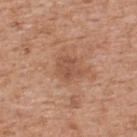Assessment: Imaged during a routine full-body skin examination; the lesion was not biopsied and no histopathology is available. Background: Automated image analysis of the tile measured a lesion color around L≈52 a*≈22 b*≈31 in CIELAB, a lesion–skin lightness drop of about 8, and a lesion-to-skin contrast of about 5.5 (normalized; higher = more distinct). It also reported a border-irregularity rating of about 3.5/10, internal color variation of about 2.5 on a 0–10 scale, and radial color variation of about 1. The software also gave a nevus-likeness score of about 0/100 and lesion-presence confidence of about 100/100. Cropped from a whole-body photographic skin survey; the tile spans about 15 mm. This is a white-light tile. The lesion is on the upper back. A male subject, roughly 60 years of age. The recorded lesion diameter is about 3 mm.